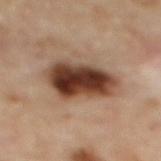Impression: This lesion was catalogued during total-body skin photography and was not selected for biopsy. Image and clinical context: The lesion is located on the upper back. A region of skin cropped from a whole-body photographic capture, roughly 15 mm wide. The subject is a female in their 60s. The lesion's longest dimension is about 6.5 mm.